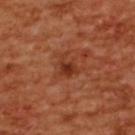A female subject aged 53 to 57.
A region of skin cropped from a whole-body photographic capture, roughly 15 mm wide.
On the upper back.